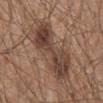biopsy_status: not biopsied; imaged during a skin examination
patient:
  sex: male
  age_approx: 65
site: abdomen
lesion_size:
  long_diameter_mm_approx: 8.0
image:
  source: total-body photography crop
  field_of_view_mm: 15
lighting: white-light